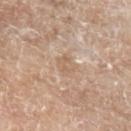This lesion was catalogued during total-body skin photography and was not selected for biopsy. Measured at roughly 2.5 mm in maximum diameter. Automated image analysis of the tile measured an outline eccentricity of about 0.7 (0 = round, 1 = elongated). It also reported a border-irregularity rating of about 2.5/10 and peripheral color asymmetry of about 0.5. A close-up tile cropped from a whole-body skin photograph, about 15 mm across. A male patient, approximately 80 years of age. The lesion is on the left upper arm.